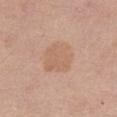Recorded during total-body skin imaging; not selected for excision or biopsy. Imaged with white-light lighting. A roughly 15 mm field-of-view crop from a total-body skin photograph. About 4 mm across. The lesion is located on the right thigh. The patient is a female aged around 55.